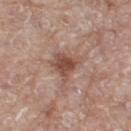Clinical impression:
Part of a total-body skin-imaging series; this lesion was reviewed on a skin check and was not flagged for biopsy.
Clinical summary:
The lesion is on the right thigh. The subject is a female in their mid- to late 70s. A 15 mm crop from a total-body photograph taken for skin-cancer surveillance. The lesion's longest dimension is about 4 mm.